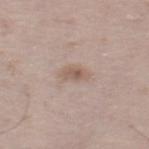  biopsy_status: not biopsied; imaged during a skin examination
  lighting: white-light
  site: right thigh
  patient:
    sex: male
    age_approx: 50
  image:
    source: total-body photography crop
    field_of_view_mm: 15